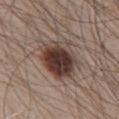| feature | finding |
|---|---|
| notes | no biopsy performed (imaged during a skin exam) |
| imaging modality | 15 mm crop, total-body photography |
| body site | the chest |
| subject | male, roughly 65 years of age |